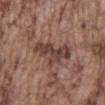Clinical impression:
The lesion was tiled from a total-body skin photograph and was not biopsied.
Clinical summary:
A male subject, about 75 years old. The lesion is on the mid back. The tile uses white-light illumination. The lesion's longest dimension is about 5 mm. A 15 mm close-up tile from a total-body photography series done for melanoma screening.